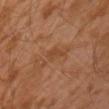The lesion's longest dimension is about 3 mm.
The lesion is located on the left upper arm.
An algorithmic analysis of the crop reported a lesion area of about 3 mm², a shape eccentricity near 0.85, and a symmetry-axis asymmetry near 0.3. The software also gave border irregularity of about 3 on a 0–10 scale, a color-variation rating of about 0.5/10, and radial color variation of about 0. The software also gave a nevus-likeness score of about 0/100 and a lesion-detection confidence of about 100/100.
This is a cross-polarized tile.
This image is a 15 mm lesion crop taken from a total-body photograph.
The patient is a male aged around 30.